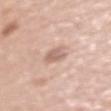Assessment:
Part of a total-body skin-imaging series; this lesion was reviewed on a skin check and was not flagged for biopsy.